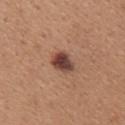Q: Was this lesion biopsied?
A: catalogued during a skin exam; not biopsied
Q: Who is the patient?
A: female, in their 30s
Q: Lesion location?
A: the upper back
Q: What kind of image is this?
A: ~15 mm crop, total-body skin-cancer survey
Q: How large is the lesion?
A: about 3.5 mm
Q: Illumination type?
A: white-light illumination